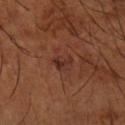Captured during whole-body skin photography for melanoma surveillance; the lesion was not biopsied.
The lesion is located on the right forearm.
A male patient, about 65 years old.
Measured at roughly 2.5 mm in maximum diameter.
A 15 mm close-up tile from a total-body photography series done for melanoma screening.